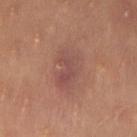Automated image analysis of the tile measured a lesion area of about 13 mm², an outline eccentricity of about 0.8 (0 = round, 1 = elongated), and a symmetry-axis asymmetry near 0.2. The analysis additionally found a mean CIELAB color near L≈49 a*≈22 b*≈24, roughly 7 lightness units darker than nearby skin, and a normalized lesion–skin contrast near 6.
A female patient about 35 years old.
Longest diameter approximately 5.5 mm.
The lesion is on the left thigh.
Cropped from a total-body skin-imaging series; the visible field is about 15 mm.